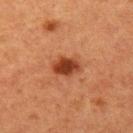| field | value |
|---|---|
| follow-up | imaged on a skin check; not biopsied |
| patient | female, aged 38–42 |
| body site | the left upper arm |
| image-analysis metrics | a border-irregularity rating of about 1.5/10, internal color variation of about 3.5 on a 0–10 scale, and radial color variation of about 1; an automated nevus-likeness rating near 100 out of 100 |
| imaging modality | ~15 mm tile from a whole-body skin photo |